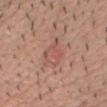follow-up = imaged on a skin check; not biopsied | image source = 15 mm crop, total-body photography | anatomic site = the chest | subject = male, in their 50s.